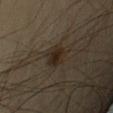biopsy status — no biopsy performed (imaged during a skin exam); TBP lesion metrics — a lesion color around L≈22 a*≈9 b*≈19 in CIELAB and roughly 7 lightness units darker than nearby skin; image — total-body-photography crop, ~15 mm field of view; lesion diameter — ~3 mm (longest diameter); patient — male, roughly 55 years of age.